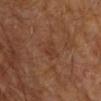Assessment: No biopsy was performed on this lesion — it was imaged during a full skin examination and was not determined to be concerning. Context: The patient is a male aged 68 to 72. The lesion is located on the left upper arm. Cropped from a total-body skin-imaging series; the visible field is about 15 mm. Imaged with cross-polarized lighting. An algorithmic analysis of the crop reported a lesion area of about 4 mm² and a symmetry-axis asymmetry near 0.4. The analysis additionally found about 5 CIELAB-L* units darker than the surrounding skin and a normalized lesion–skin contrast near 5. And it measured a border-irregularity index near 4/10 and peripheral color asymmetry of about 0.5. And it measured an automated nevus-likeness rating near 0 out of 100 and lesion-presence confidence of about 100/100.